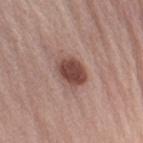{"biopsy_status": "not biopsied; imaged during a skin examination", "lesion_size": {"long_diameter_mm_approx": 3.5}, "patient": {"sex": "male", "age_approx": 65}, "image": {"source": "total-body photography crop", "field_of_view_mm": 15}, "site": "arm", "lighting": "white-light"}